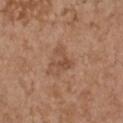{"biopsy_status": "not biopsied; imaged during a skin examination", "image": {"source": "total-body photography crop", "field_of_view_mm": 15}, "site": "chest", "lesion_size": {"long_diameter_mm_approx": 3.5}, "patient": {"sex": "female", "age_approx": 65}}